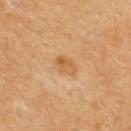biopsy_status: not biopsied; imaged during a skin examination
automated_metrics:
  area_mm2_approx: 4.5
  eccentricity: 0.75
  cielab_L: 49
  cielab_a: 18
  cielab_b: 36
  vs_skin_darker_L: 7.0
  vs_skin_contrast_norm: 6.5
  nevus_likeness_0_100: 15
site: upper back
lighting: cross-polarized
image:
  source: total-body photography crop
  field_of_view_mm: 15
patient:
  sex: female
  age_approx: 55
lesion_size:
  long_diameter_mm_approx: 3.0